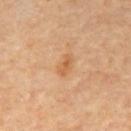biopsy status — total-body-photography surveillance lesion; no biopsy
image source — 15 mm crop, total-body photography
subject — male, aged around 65
illumination — cross-polarized
location — the mid back
size — ~3 mm (longest diameter)
automated metrics — a lesion color around L≈57 a*≈21 b*≈38 in CIELAB, about 8 CIELAB-L* units darker than the surrounding skin, and a normalized lesion–skin contrast near 6; a border-irregularity index near 3/10, internal color variation of about 1.5 on a 0–10 scale, and peripheral color asymmetry of about 0.5